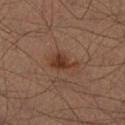Captured during whole-body skin photography for melanoma surveillance; the lesion was not biopsied. A male patient aged 33–37. The lesion is located on the left leg. This image is a 15 mm lesion crop taken from a total-body photograph.